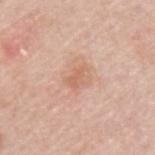workup = no biopsy performed (imaged during a skin exam)
subject = male, aged around 60
lighting = white-light
acquisition = 15 mm crop, total-body photography
size = ≈2.5 mm
location = the upper back